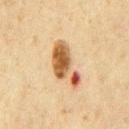Clinical impression: The lesion was photographed on a routine skin check and not biopsied; there is no pathology result. Context: About 6 mm across. The patient is a male aged around 60. A close-up tile cropped from a whole-body skin photograph, about 15 mm across. From the chest.